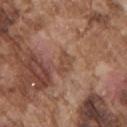Captured during whole-body skin photography for melanoma surveillance; the lesion was not biopsied. Captured under white-light illumination. The patient is a male aged 73 to 77. On the chest. Automated tile analysis of the lesion measured an eccentricity of roughly 0.85 and two-axis asymmetry of about 0.4. The analysis additionally found border irregularity of about 5 on a 0–10 scale, a within-lesion color-variation index near 0/10, and radial color variation of about 0. The lesion's longest dimension is about 2.5 mm. A region of skin cropped from a whole-body photographic capture, roughly 15 mm wide.